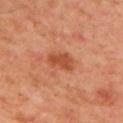Recorded during total-body skin imaging; not selected for excision or biopsy. Approximately 3.5 mm at its widest. Cropped from a whole-body photographic skin survey; the tile spans about 15 mm. On the left arm. A male patient roughly 50 years of age. An algorithmic analysis of the crop reported an average lesion color of about L≈51 a*≈30 b*≈38 (CIELAB), a lesion–skin lightness drop of about 10, and a normalized border contrast of about 7.5. Imaged with cross-polarized lighting.